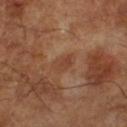The lesion was photographed on a routine skin check and not biopsied; there is no pathology result. Imaged with cross-polarized lighting. The total-body-photography lesion software estimated an area of roughly 4 mm², a shape eccentricity near 0.75, and two-axis asymmetry of about 0.25. The software also gave a nevus-likeness score of about 0/100. Measured at roughly 3 mm in maximum diameter. A 15 mm close-up extracted from a 3D total-body photography capture. A male subject aged 63 to 67.